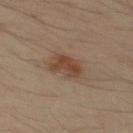biopsy status: imaged on a skin check; not biopsied
site: the back
TBP lesion metrics: a footprint of about 8 mm² and an eccentricity of roughly 0.65; border irregularity of about 2.5 on a 0–10 scale, a within-lesion color-variation index near 3.5/10, and radial color variation of about 1
lesion size: ≈3.5 mm
lighting: cross-polarized illumination
patient: male, roughly 50 years of age
image source: ~15 mm tile from a whole-body skin photo